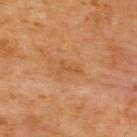Impression:
Imaged during a routine full-body skin examination; the lesion was not biopsied and no histopathology is available.
Image and clinical context:
From the upper back. About 3 mm across. Imaged with cross-polarized lighting. The subject is a male in their 60s. An algorithmic analysis of the crop reported a lesion area of about 2.5 mm² and an eccentricity of roughly 0.9. It also reported a mean CIELAB color near L≈52 a*≈24 b*≈40. A lesion tile, about 15 mm wide, cut from a 3D total-body photograph.